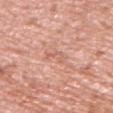This lesion was catalogued during total-body skin photography and was not selected for biopsy. A male patient approximately 70 years of age. A 15 mm close-up tile from a total-body photography series done for melanoma screening. On the arm. The lesion's longest dimension is about 3 mm.